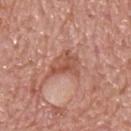Impression:
The lesion was photographed on a routine skin check and not biopsied; there is no pathology result.
Acquisition and patient details:
Approximately 3.5 mm at its widest. A male patient aged around 75. The tile uses white-light illumination. The total-body-photography lesion software estimated a mean CIELAB color near L≈53 a*≈25 b*≈30, roughly 9 lightness units darker than nearby skin, and a normalized border contrast of about 6.5. The lesion is on the upper back. Cropped from a whole-body photographic skin survey; the tile spans about 15 mm.